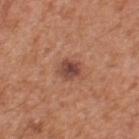Assessment: Recorded during total-body skin imaging; not selected for excision or biopsy. Clinical summary: Automated image analysis of the tile measured a footprint of about 5 mm² and two-axis asymmetry of about 0.2. The software also gave an average lesion color of about L≈45 a*≈24 b*≈27 (CIELAB), a lesion–skin lightness drop of about 12, and a normalized border contrast of about 9. And it measured a nevus-likeness score of about 70/100 and a detector confidence of about 100 out of 100 that the crop contains a lesion. The subject is a male aged 53 to 57. The lesion is on the upper back. Cropped from a whole-body photographic skin survey; the tile spans about 15 mm. Approximately 2.5 mm at its widest.